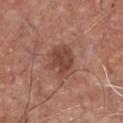<tbp_lesion>
<biopsy_status>not biopsied; imaged during a skin examination</biopsy_status>
<patient>
  <sex>male</sex>
  <age_approx>65</age_approx>
</patient>
<lesion_size>
  <long_diameter_mm_approx>4.0</long_diameter_mm_approx>
</lesion_size>
<lighting>white-light</lighting>
<image>
  <source>total-body photography crop</source>
  <field_of_view_mm>15</field_of_view_mm>
</image>
<site>front of the torso</site>
</tbp_lesion>